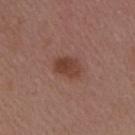Q: What are the patient's age and sex?
A: female, roughly 45 years of age
Q: How was the tile lit?
A: white-light illumination
Q: What kind of image is this?
A: ~15 mm crop, total-body skin-cancer survey
Q: Lesion location?
A: the arm
Q: Automated lesion metrics?
A: an automated nevus-likeness rating near 80 out of 100 and lesion-presence confidence of about 100/100